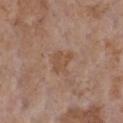Recorded during total-body skin imaging; not selected for excision or biopsy.
Automated image analysis of the tile measured a footprint of about 5.5 mm², an eccentricity of roughly 0.6, and a shape-asymmetry score of about 0.3 (0 = symmetric). It also reported a lesion color around L≈50 a*≈18 b*≈30 in CIELAB, about 6 CIELAB-L* units darker than the surrounding skin, and a normalized lesion–skin contrast near 5.5. The analysis additionally found border irregularity of about 3 on a 0–10 scale and peripheral color asymmetry of about 0.5. The analysis additionally found a classifier nevus-likeness of about 0/100 and lesion-presence confidence of about 100/100.
Measured at roughly 3 mm in maximum diameter.
A female patient, aged around 85 or older.
This is a white-light tile.
A 15 mm close-up tile from a total-body photography series done for melanoma screening.
Located on the chest.